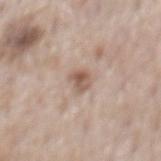Q: Was this lesion biopsied?
A: no biopsy performed (imaged during a skin exam)